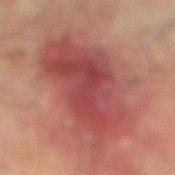Impression: Imaged during a routine full-body skin examination; the lesion was not biopsied and no histopathology is available. Context: Approximately 9.5 mm at its widest. A 15 mm close-up tile from a total-body photography series done for melanoma screening. The patient is a male about 75 years old. Captured under cross-polarized illumination. The lesion is on the right lower leg.